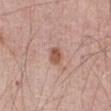No biopsy was performed on this lesion — it was imaged during a full skin examination and was not determined to be concerning.
Automated tile analysis of the lesion measured a lesion area of about 4 mm², an outline eccentricity of about 0.6 (0 = round, 1 = elongated), and two-axis asymmetry of about 0.2. The software also gave a border-irregularity index near 2/10, a color-variation rating of about 3/10, and a peripheral color-asymmetry measure near 1. The software also gave a nevus-likeness score of about 85/100 and a detector confidence of about 100 out of 100 that the crop contains a lesion.
Imaged with white-light lighting.
The lesion is located on the abdomen.
A male subject, aged approximately 80.
Longest diameter approximately 2.5 mm.
Cropped from a total-body skin-imaging series; the visible field is about 15 mm.